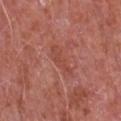| field | value |
|---|---|
| location | the chest |
| imaging modality | ~15 mm tile from a whole-body skin photo |
| lighting | white-light illumination |
| subject | male, about 65 years old |
| TBP lesion metrics | a footprint of about 6 mm² and two-axis asymmetry of about 0.35; a lesion color around L≈48 a*≈28 b*≈29 in CIELAB, roughly 6 lightness units darker than nearby skin, and a lesion-to-skin contrast of about 5 (normalized; higher = more distinct); border irregularity of about 4 on a 0–10 scale and peripheral color asymmetry of about 0.5; a classifier nevus-likeness of about 0/100 and a detector confidence of about 90 out of 100 that the crop contains a lesion |